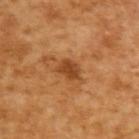The lesion was tiled from a total-body skin photograph and was not biopsied.
Cropped from a whole-body photographic skin survey; the tile spans about 15 mm.
The tile uses cross-polarized illumination.
The recorded lesion diameter is about 3 mm.
The lesion-visualizer software estimated an area of roughly 4.5 mm² and a shape-asymmetry score of about 0.3 (0 = symmetric). It also reported internal color variation of about 1.5 on a 0–10 scale and a peripheral color-asymmetry measure near 0.5. The software also gave a nevus-likeness score of about 85/100 and lesion-presence confidence of about 100/100.
A male subject aged 63–67.